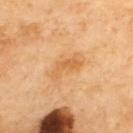Assessment: Part of a total-body skin-imaging series; this lesion was reviewed on a skin check and was not flagged for biopsy.